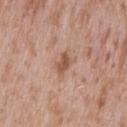No biopsy was performed on this lesion — it was imaged during a full skin examination and was not determined to be concerning. A lesion tile, about 15 mm wide, cut from a 3D total-body photograph. The lesion is located on the lower back. A male patient in their mid-40s.